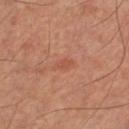Q: Is there a histopathology result?
A: total-body-photography surveillance lesion; no biopsy
Q: What is the imaging modality?
A: 15 mm crop, total-body photography
Q: How was the tile lit?
A: cross-polarized
Q: What is the anatomic site?
A: the left lower leg
Q: Automated lesion metrics?
A: a footprint of about 2.5 mm², an eccentricity of roughly 0.85, and two-axis asymmetry of about 0.3
Q: Patient demographics?
A: male, roughly 55 years of age